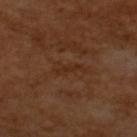Recorded during total-body skin imaging; not selected for excision or biopsy.
Cropped from a total-body skin-imaging series; the visible field is about 15 mm.
A male subject in their mid- to late 60s.